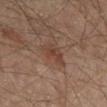follow-up: no biopsy performed (imaged during a skin exam)
diameter: ≈3 mm
subject: male, aged 63 to 67
acquisition: ~15 mm crop, total-body skin-cancer survey
illumination: cross-polarized illumination
site: the left thigh
automated lesion analysis: a footprint of about 5 mm², an eccentricity of roughly 0.7, and a shape-asymmetry score of about 0.3 (0 = symmetric); a lesion–skin lightness drop of about 7 and a normalized lesion–skin contrast near 6.5; a classifier nevus-likeness of about 40/100